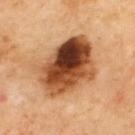Imaged during a routine full-body skin examination; the lesion was not biopsied and no histopathology is available.
A 15 mm close-up extracted from a 3D total-body photography capture.
The lesion's longest dimension is about 8 mm.
This is a cross-polarized tile.
On the upper back.